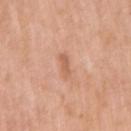* workup: total-body-photography surveillance lesion; no biopsy
* lesion diameter: ≈2.5 mm
* subject: female, aged 63–67
* imaging modality: ~15 mm tile from a whole-body skin photo
* automated metrics: a border-irregularity rating of about 3/10 and radial color variation of about 0
* location: the arm
* lighting: white-light illumination